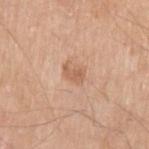{
  "biopsy_status": "not biopsied; imaged during a skin examination",
  "patient": {
    "sex": "male",
    "age_approx": 70
  },
  "lighting": "white-light",
  "site": "left upper arm",
  "lesion_size": {
    "long_diameter_mm_approx": 2.5
  },
  "image": {
    "source": "total-body photography crop",
    "field_of_view_mm": 15
  }
}